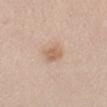Q: Was this lesion biopsied?
A: no biopsy performed (imaged during a skin exam)
Q: Illumination type?
A: white-light
Q: Patient demographics?
A: female, aged 23 to 27
Q: What is the imaging modality?
A: ~15 mm tile from a whole-body skin photo
Q: What did automated image analysis measure?
A: an area of roughly 5.5 mm² and an eccentricity of roughly 0.5; an average lesion color of about L≈63 a*≈18 b*≈30 (CIELAB) and a normalized border contrast of about 6
Q: Where on the body is the lesion?
A: the left forearm
Q: What is the lesion's diameter?
A: ~2.5 mm (longest diameter)